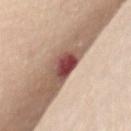Impression: The lesion was tiled from a total-body skin photograph and was not biopsied. Context: A close-up tile cropped from a whole-body skin photograph, about 15 mm across. A female patient, aged approximately 70. The total-body-photography lesion software estimated an area of roughly 5.5 mm² and two-axis asymmetry of about 0.4. The software also gave a border-irregularity rating of about 4/10, internal color variation of about 3.5 on a 0–10 scale, and radial color variation of about 1. Located on the mid back. Approximately 4 mm at its widest.